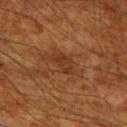Recorded during total-body skin imaging; not selected for excision or biopsy.
The lesion is located on the right upper arm.
Automated tile analysis of the lesion measured roughly 6 lightness units darker than nearby skin.
The tile uses cross-polarized illumination.
The patient is a male in their mid- to late 60s.
Longest diameter approximately 3 mm.
A 15 mm close-up tile from a total-body photography series done for melanoma screening.